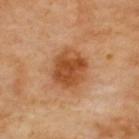notes = imaged on a skin check; not biopsied
image-analysis metrics = lesion-presence confidence of about 100/100
site = the back
lesion size = about 4.5 mm
imaging modality = ~15 mm crop, total-body skin-cancer survey